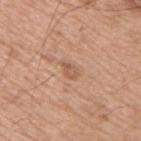Assessment:
The lesion was photographed on a routine skin check and not biopsied; there is no pathology result.
Context:
Imaged with white-light lighting. The lesion is on the upper back. A 15 mm close-up extracted from a 3D total-body photography capture. Automated tile analysis of the lesion measured an area of roughly 3 mm², an eccentricity of roughly 0.8, and a shape-asymmetry score of about 0.3 (0 = symmetric). The software also gave roughly 8 lightness units darker than nearby skin and a lesion-to-skin contrast of about 5.5 (normalized; higher = more distinct). The software also gave a classifier nevus-likeness of about 0/100 and a lesion-detection confidence of about 100/100. A male subject, about 55 years old. Longest diameter approximately 2.5 mm.